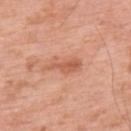<tbp_lesion>
<biopsy_status>not biopsied; imaged during a skin examination</biopsy_status>
<patient>
  <sex>male</sex>
  <age_approx>60</age_approx>
</patient>
<lesion_size>
  <long_diameter_mm_approx>4.0</long_diameter_mm_approx>
</lesion_size>
<image>
  <source>total-body photography crop</source>
  <field_of_view_mm>15</field_of_view_mm>
</image>
<lighting>white-light</lighting>
<site>upper back</site>
</tbp_lesion>